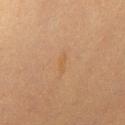{
  "image": {
    "source": "total-body photography crop",
    "field_of_view_mm": 15
  },
  "site": "chest",
  "patient": {
    "sex": "female",
    "age_approx": 55
  },
  "lighting": "cross-polarized"
}